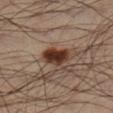The lesion was photographed on a routine skin check and not biopsied; there is no pathology result.
On the right lower leg.
Imaged with cross-polarized lighting.
A male subject aged 33–37.
A lesion tile, about 15 mm wide, cut from a 3D total-body photograph.
Automated tile analysis of the lesion measured a border-irregularity index near 2/10 and radial color variation of about 1. And it measured an automated nevus-likeness rating near 100 out of 100 and lesion-presence confidence of about 100/100.